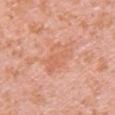Impression: Recorded during total-body skin imaging; not selected for excision or biopsy. Acquisition and patient details: The patient is a female aged 38–42. Measured at roughly 5 mm in maximum diameter. Automated image analysis of the tile measured an area of roughly 9 mm², an eccentricity of roughly 0.9, and a symmetry-axis asymmetry near 0.3. The software also gave a color-variation rating of about 3/10 and a peripheral color-asymmetry measure near 1. The analysis additionally found an automated nevus-likeness rating near 0 out of 100 and a lesion-detection confidence of about 100/100. Cropped from a whole-body photographic skin survey; the tile spans about 15 mm. On the chest.